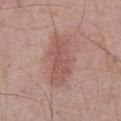Assessment: The lesion was photographed on a routine skin check and not biopsied; there is no pathology result. Acquisition and patient details: The tile uses white-light illumination. From the abdomen. A region of skin cropped from a whole-body photographic capture, roughly 15 mm wide. The patient is a male aged around 70. The recorded lesion diameter is about 6 mm.